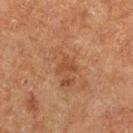biopsy status: total-body-photography surveillance lesion; no biopsy | imaging modality: ~15 mm crop, total-body skin-cancer survey | body site: the leg | patient: male, in their mid-60s.